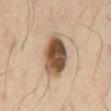Clinical impression: Recorded during total-body skin imaging; not selected for excision or biopsy. Context: A close-up tile cropped from a whole-body skin photograph, about 15 mm across. Approximately 6 mm at its widest. From the chest. A male patient, aged 38–42. This is a cross-polarized tile. Automated image analysis of the tile measured an eccentricity of roughly 0.8. It also reported a mean CIELAB color near L≈51 a*≈15 b*≈30 and a lesion-to-skin contrast of about 12 (normalized; higher = more distinct). And it measured border irregularity of about 1.5 on a 0–10 scale, a color-variation rating of about 8.5/10, and radial color variation of about 3.